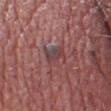Q: Was this lesion biopsied?
A: total-body-photography surveillance lesion; no biopsy
Q: What is the lesion's diameter?
A: about 3.5 mm
Q: Patient demographics?
A: male, aged around 60
Q: What did automated image analysis measure?
A: an area of roughly 5.5 mm²; a lesion–skin lightness drop of about 7 and a lesion-to-skin contrast of about 6 (normalized; higher = more distinct); border irregularity of about 3.5 on a 0–10 scale, a color-variation rating of about 5.5/10, and radial color variation of about 2; an automated nevus-likeness rating near 0 out of 100 and a lesion-detection confidence of about 80/100
Q: How was the tile lit?
A: white-light
Q: Where on the body is the lesion?
A: the head or neck
Q: What kind of image is this?
A: total-body-photography crop, ~15 mm field of view